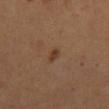{
  "biopsy_status": "not biopsied; imaged during a skin examination",
  "site": "mid back",
  "image": {
    "source": "total-body photography crop",
    "field_of_view_mm": 15
  },
  "lesion_size": {
    "long_diameter_mm_approx": 2.0
  },
  "patient": {
    "sex": "female"
  }
}